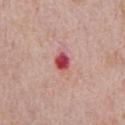No biopsy was performed on this lesion — it was imaged during a full skin examination and was not determined to be concerning. Imaged with white-light lighting. The lesion is on the front of the torso. This image is a 15 mm lesion crop taken from a total-body photograph. A male subject, approximately 70 years of age.